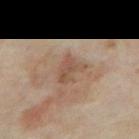{
  "biopsy_status": "not biopsied; imaged during a skin examination",
  "automated_metrics": {
    "vs_skin_darker_L": 8.0,
    "vs_skin_contrast_norm": 6.0,
    "nevus_likeness_0_100": 0,
    "lesion_detection_confidence_0_100": 100
  },
  "site": "arm",
  "lesion_size": {
    "long_diameter_mm_approx": 3.0
  },
  "patient": {
    "sex": "female",
    "age_approx": 35
  },
  "image": {
    "source": "total-body photography crop",
    "field_of_view_mm": 15
  }
}